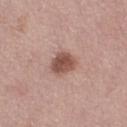Clinical impression:
No biopsy was performed on this lesion — it was imaged during a full skin examination and was not determined to be concerning.
Image and clinical context:
A female patient, aged 48–52. A roughly 15 mm field-of-view crop from a total-body skin photograph. Located on the right thigh. Imaged with white-light lighting.